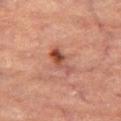Context: An algorithmic analysis of the crop reported an average lesion color of about L≈41 a*≈23 b*≈26 (CIELAB), about 10 CIELAB-L* units darker than the surrounding skin, and a normalized border contrast of about 8. And it measured a border-irregularity rating of about 5/10. The analysis additionally found a classifier nevus-likeness of about 20/100 and a lesion-detection confidence of about 100/100. Cropped from a total-body skin-imaging series; the visible field is about 15 mm. A female patient about 70 years old. Longest diameter approximately 4 mm. The lesion is on the leg. Captured under cross-polarized illumination.